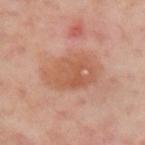Findings:
• notes: catalogued during a skin exam; not biopsied
• image: ~15 mm crop, total-body skin-cancer survey
• illumination: cross-polarized illumination
• lesion size: about 6 mm
• TBP lesion metrics: a lesion area of about 18 mm² and an outline eccentricity of about 0.8 (0 = round, 1 = elongated); a lesion color around L≈56 a*≈23 b*≈32 in CIELAB, a lesion–skin lightness drop of about 8, and a normalized border contrast of about 6.5; border irregularity of about 2 on a 0–10 scale, a color-variation rating of about 3.5/10, and radial color variation of about 1
• anatomic site: the left upper arm
• subject: male, aged 48–52